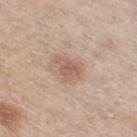Part of a total-body skin-imaging series; this lesion was reviewed on a skin check and was not flagged for biopsy.
A female subject, approximately 65 years of age.
The lesion is located on the right thigh.
The recorded lesion diameter is about 2.5 mm.
A close-up tile cropped from a whole-body skin photograph, about 15 mm across.